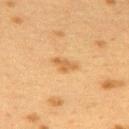Captured during whole-body skin photography for melanoma surveillance; the lesion was not biopsied. Approximately 3 mm at its widest. The lesion is located on the upper back. The tile uses cross-polarized illumination. The subject is a female aged around 40. This image is a 15 mm lesion crop taken from a total-body photograph.